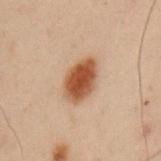This lesion was catalogued during total-body skin photography and was not selected for biopsy. The total-body-photography lesion software estimated a lesion–skin lightness drop of about 14 and a normalized lesion–skin contrast near 11.5. Approximately 4.5 mm at its widest. The lesion is located on the left upper arm. A male subject, roughly 55 years of age. A roughly 15 mm field-of-view crop from a total-body skin photograph.